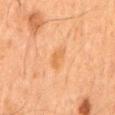Clinical impression:
Imaged during a routine full-body skin examination; the lesion was not biopsied and no histopathology is available.
Image and clinical context:
From the mid back. Captured under cross-polarized illumination. A close-up tile cropped from a whole-body skin photograph, about 15 mm across. The patient is a male aged approximately 65. The recorded lesion diameter is about 3 mm. The total-body-photography lesion software estimated an average lesion color of about L≈54 a*≈21 b*≈38 (CIELAB) and a normalized border contrast of about 5.5.